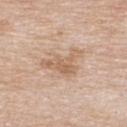Captured during whole-body skin photography for melanoma surveillance; the lesion was not biopsied. The subject is a male aged around 85 or older. The lesion is located on the upper back. Captured under white-light illumination. A region of skin cropped from a whole-body photographic capture, roughly 15 mm wide. Measured at roughly 5 mm in maximum diameter. An algorithmic analysis of the crop reported about 9 CIELAB-L* units darker than the surrounding skin and a normalized border contrast of about 6.5. And it measured a nevus-likeness score of about 0/100 and a lesion-detection confidence of about 100/100.